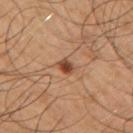notes: catalogued during a skin exam; not biopsied | patient: male, aged 48–52 | automated metrics: an area of roughly 3 mm², a shape eccentricity near 0.85, and two-axis asymmetry of about 0.3; a lesion color around L≈34 a*≈18 b*≈26 in CIELAB, about 10 CIELAB-L* units darker than the surrounding skin, and a normalized border contrast of about 9; a border-irregularity index near 2.5/10, internal color variation of about 3 on a 0–10 scale, and peripheral color asymmetry of about 1; a nevus-likeness score of about 90/100 | diameter: ≈2.5 mm | image source: 15 mm crop, total-body photography | anatomic site: the right upper arm.